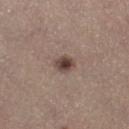This lesion was catalogued during total-body skin photography and was not selected for biopsy.
An algorithmic analysis of the crop reported an average lesion color of about L≈43 a*≈15 b*≈21 (CIELAB) and a lesion–skin lightness drop of about 13. The analysis additionally found a lesion-detection confidence of about 100/100.
A female patient aged approximately 65.
This is a white-light tile.
From the right thigh.
A close-up tile cropped from a whole-body skin photograph, about 15 mm across.
Measured at roughly 2.5 mm in maximum diameter.